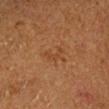  biopsy_status: not biopsied; imaged during a skin examination
  automated_metrics:
    area_mm2_approx: 3.0
    eccentricity: 0.9
    cielab_L: 36
    cielab_a: 19
    cielab_b: 31
    vs_skin_darker_L: 4.0
    vs_skin_contrast_norm: 4.5
    border_irregularity_0_10: 6.0
    color_variation_0_10: 0.0
  site: head or neck
  patient:
    sex: male
    age_approx: 60
  image:
    source: total-body photography crop
    field_of_view_mm: 15
  lesion_size:
    long_diameter_mm_approx: 3.0
  lighting: cross-polarized